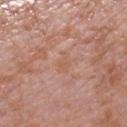Assessment: Part of a total-body skin-imaging series; this lesion was reviewed on a skin check and was not flagged for biopsy. Context: The tile uses white-light illumination. Measured at roughly 3 mm in maximum diameter. A 15 mm crop from a total-body photograph taken for skin-cancer surveillance. A female patient in their 40s. On the right lower leg.